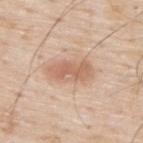Clinical impression:
Captured during whole-body skin photography for melanoma surveillance; the lesion was not biopsied.
Context:
A male subject roughly 80 years of age. The recorded lesion diameter is about 5 mm. A close-up tile cropped from a whole-body skin photograph, about 15 mm across. The lesion-visualizer software estimated an area of roughly 11 mm², an eccentricity of roughly 0.85, and a symmetry-axis asymmetry near 0.2. It also reported a lesion color around L≈63 a*≈20 b*≈31 in CIELAB, about 10 CIELAB-L* units darker than the surrounding skin, and a normalized lesion–skin contrast near 6.5. The analysis additionally found a classifier nevus-likeness of about 85/100. The tile uses white-light illumination. The lesion is on the upper back.